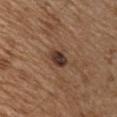Impression: The lesion was photographed on a routine skin check and not biopsied; there is no pathology result. Background: Measured at roughly 2.5 mm in maximum diameter. A 15 mm crop from a total-body photograph taken for skin-cancer surveillance. A male subject aged 68–72. Located on the chest. Captured under white-light illumination.